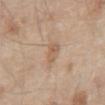• follow-up — no biopsy performed (imaged during a skin exam)
• illumination — white-light
• acquisition — total-body-photography crop, ~15 mm field of view
• lesion diameter — ~2.5 mm (longest diameter)
• body site — the front of the torso
• image-analysis metrics — a shape eccentricity near 0.8 and a symmetry-axis asymmetry near 0.4; a classifier nevus-likeness of about 0/100 and lesion-presence confidence of about 100/100
• subject — male, aged 78 to 82